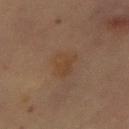Impression: No biopsy was performed on this lesion — it was imaged during a full skin examination and was not determined to be concerning. Context: This is a cross-polarized tile. Cropped from a total-body skin-imaging series; the visible field is about 15 mm. The patient is a female in their 60s. From the abdomen. Automated image analysis of the tile measured an average lesion color of about L≈36 a*≈15 b*≈27 (CIELAB), about 5 CIELAB-L* units darker than the surrounding skin, and a normalized border contrast of about 6. It also reported a classifier nevus-likeness of about 15/100 and a detector confidence of about 100 out of 100 that the crop contains a lesion.